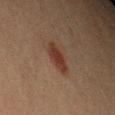Case summary:
• follow-up — imaged on a skin check; not biopsied
• site — the left upper arm
• patient — male, aged 38–42
• automated lesion analysis — a mean CIELAB color near L≈28 a*≈18 b*≈23, roughly 8 lightness units darker than nearby skin, and a lesion-to-skin contrast of about 8.5 (normalized; higher = more distinct); radial color variation of about 1
• image source — ~15 mm crop, total-body skin-cancer survey
• size — ~4 mm (longest diameter)